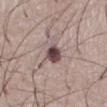Findings:
* follow-up: catalogued during a skin exam; not biopsied
* anatomic site: the abdomen
* imaging modality: 15 mm crop, total-body photography
* subject: male, in their mid- to late 50s